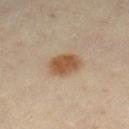The lesion was tiled from a total-body skin photograph and was not biopsied.
The recorded lesion diameter is about 4 mm.
On the right lower leg.
A female subject aged 43–47.
The tile uses cross-polarized illumination.
Cropped from a whole-body photographic skin survey; the tile spans about 15 mm.